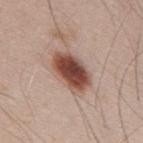<case>
  <biopsy_status>not biopsied; imaged during a skin examination</biopsy_status>
  <automated_metrics>
    <area_mm2_approx>14.0</area_mm2_approx>
    <eccentricity>0.8</eccentricity>
    <shape_asymmetry>0.15</shape_asymmetry>
    <cielab_L>48</cielab_L>
    <cielab_a>21</cielab_a>
    <cielab_b>26</cielab_b>
    <vs_skin_darker_L>18.0</vs_skin_darker_L>
    <vs_skin_contrast_norm>12.0</vs_skin_contrast_norm>
    <border_irregularity_0_10>1.5</border_irregularity_0_10>
    <color_variation_0_10>7.0</color_variation_0_10>
    <peripheral_color_asymmetry>2.0</peripheral_color_asymmetry>
  </automated_metrics>
  <site>mid back</site>
  <image>
    <source>total-body photography crop</source>
    <field_of_view_mm>15</field_of_view_mm>
  </image>
  <lighting>white-light</lighting>
  <patient>
    <sex>male</sex>
    <age_approx>35</age_approx>
  </patient>
</case>